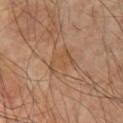Assessment: This lesion was catalogued during total-body skin photography and was not selected for biopsy. Clinical summary: A male patient aged 58–62. Automated image analysis of the tile measured a lesion area of about 7 mm², a shape eccentricity near 0.8, and a shape-asymmetry score of about 0.4 (0 = symmetric). The software also gave a lesion-to-skin contrast of about 5 (normalized; higher = more distinct). It also reported a border-irregularity index near 5.5/10 and a peripheral color-asymmetry measure near 0.5. And it measured an automated nevus-likeness rating near 0 out of 100 and lesion-presence confidence of about 100/100. The lesion is on the left upper arm. Longest diameter approximately 4 mm. A region of skin cropped from a whole-body photographic capture, roughly 15 mm wide.